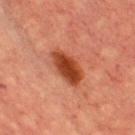Findings:
- anatomic site · the chest
- image source · total-body-photography crop, ~15 mm field of view
- tile lighting · cross-polarized
- patient · male, in their 60s
- TBP lesion metrics · an automated nevus-likeness rating near 100 out of 100 and a lesion-detection confidence of about 100/100
- diameter · ~4.5 mm (longest diameter)